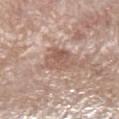Q: Was a biopsy performed?
A: imaged on a skin check; not biopsied
Q: Patient demographics?
A: male, aged approximately 70
Q: Illumination type?
A: white-light illumination
Q: Lesion size?
A: about 4 mm
Q: What is the imaging modality?
A: ~15 mm crop, total-body skin-cancer survey
Q: What did automated image analysis measure?
A: an area of roughly 9.5 mm², an outline eccentricity of about 0.5 (0 = round, 1 = elongated), and two-axis asymmetry of about 0.3; a mean CIELAB color near L≈57 a*≈18 b*≈26, about 9 CIELAB-L* units darker than the surrounding skin, and a lesion-to-skin contrast of about 6.5 (normalized; higher = more distinct); a classifier nevus-likeness of about 0/100 and a detector confidence of about 100 out of 100 that the crop contains a lesion
Q: What is the anatomic site?
A: the left lower leg